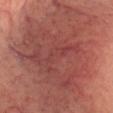| key | value |
|---|---|
| follow-up | catalogued during a skin exam; not biopsied |
| tile lighting | cross-polarized |
| diameter | ≈20.5 mm |
| anatomic site | the head or neck |
| image-analysis metrics | a footprint of about 105 mm², an outline eccentricity of about 0.9 (0 = round, 1 = elongated), and two-axis asymmetry of about 0.35; a lesion color around L≈45 a*≈26 b*≈24 in CIELAB and a normalized lesion–skin contrast near 7.5; lesion-presence confidence of about 75/100 |
| subject | male, aged approximately 70 |
| image | ~15 mm crop, total-body skin-cancer survey |